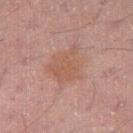Impression: No biopsy was performed on this lesion — it was imaged during a full skin examination and was not determined to be concerning. Context: A male subject, in their 50s. From the right thigh. Longest diameter approximately 4.5 mm. This image is a 15 mm lesion crop taken from a total-body photograph. Imaged with cross-polarized lighting.